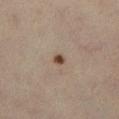The lesion was photographed on a routine skin check and not biopsied; there is no pathology result.
A 15 mm close-up extracted from a 3D total-body photography capture.
A female subject about 45 years old.
The lesion-visualizer software estimated a footprint of about 2 mm² and a shape-asymmetry score of about 0.25 (0 = symmetric). The software also gave an average lesion color of about L≈38 a*≈14 b*≈24 (CIELAB), about 12 CIELAB-L* units darker than the surrounding skin, and a lesion-to-skin contrast of about 10.5 (normalized; higher = more distinct). The analysis additionally found a classifier nevus-likeness of about 95/100.
Located on the left lower leg.
The lesion's longest dimension is about 1.5 mm.
Captured under cross-polarized illumination.